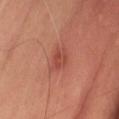follow-up=imaged on a skin check; not biopsied
illumination=cross-polarized
body site=the right thigh
patient=about 65 years old
acquisition=~15 mm tile from a whole-body skin photo